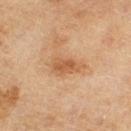| field | value |
|---|---|
| biopsy status | total-body-photography surveillance lesion; no biopsy |
| patient | female, aged 58 to 62 |
| image | ~15 mm tile from a whole-body skin photo |
| tile lighting | cross-polarized illumination |
| body site | the right thigh |
| lesion size | about 4 mm |